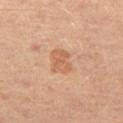| key | value |
|---|---|
| workup | catalogued during a skin exam; not biopsied |
| body site | the left thigh |
| TBP lesion metrics | a lesion color around L≈58 a*≈22 b*≈34 in CIELAB and about 8 CIELAB-L* units darker than the surrounding skin |
| patient | female, aged 43 to 47 |
| imaging modality | ~15 mm tile from a whole-body skin photo |